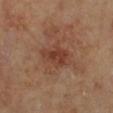<tbp_lesion>
<biopsy_status>not biopsied; imaged during a skin examination</biopsy_status>
<automated_metrics>
  <area_mm2_approx>6.5</area_mm2_approx>
  <cielab_L>38</cielab_L>
  <cielab_a>22</cielab_a>
  <cielab_b>28</cielab_b>
  <vs_skin_darker_L>8.0</vs_skin_darker_L>
  <vs_skin_contrast_norm>7.0</vs_skin_contrast_norm>
  <nevus_likeness_0_100>10</nevus_likeness_0_100>
  <lesion_detection_confidence_0_100>100</lesion_detection_confidence_0_100>
</automated_metrics>
<lighting>cross-polarized</lighting>
<image>
  <source>total-body photography crop</source>
  <field_of_view_mm>15</field_of_view_mm>
</image>
<site>right lower leg</site>
<lesion_size>
  <long_diameter_mm_approx>3.5</long_diameter_mm_approx>
</lesion_size>
<patient>
  <sex>male</sex>
  <age_approx>70</age_approx>
</patient>
</tbp_lesion>